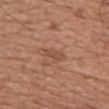Assessment: This lesion was catalogued during total-body skin photography and was not selected for biopsy. Context: Located on the chest. The tile uses white-light illumination. A 15 mm close-up extracted from a 3D total-body photography capture. An algorithmic analysis of the crop reported two-axis asymmetry of about 0.3. It also reported a mean CIELAB color near L≈50 a*≈22 b*≈31. The subject is a male approximately 55 years of age.